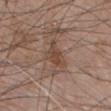| key | value |
|---|---|
| biopsy status | catalogued during a skin exam; not biopsied |
| tile lighting | white-light |
| anatomic site | the chest |
| automated lesion analysis | an average lesion color of about L≈45 a*≈18 b*≈26 (CIELAB), about 8 CIELAB-L* units darker than the surrounding skin, and a normalized border contrast of about 6.5; internal color variation of about 1.5 on a 0–10 scale and peripheral color asymmetry of about 0.5 |
| lesion size | about 3.5 mm |
| image | total-body-photography crop, ~15 mm field of view |
| patient | male, aged 68–72 |